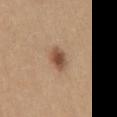This lesion was catalogued during total-body skin photography and was not selected for biopsy.
A female patient, aged approximately 35.
The total-body-photography lesion software estimated a lesion area of about 5.5 mm², an outline eccentricity of about 0.75 (0 = round, 1 = elongated), and two-axis asymmetry of about 0.25. The analysis additionally found border irregularity of about 2 on a 0–10 scale, internal color variation of about 5 on a 0–10 scale, and a peripheral color-asymmetry measure near 1.5. It also reported a classifier nevus-likeness of about 95/100 and lesion-presence confidence of about 100/100.
The lesion's longest dimension is about 3 mm.
The lesion is located on the mid back.
Cropped from a total-body skin-imaging series; the visible field is about 15 mm.
Captured under white-light illumination.